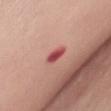biopsy status: catalogued during a skin exam; not biopsied
patient: female, in their mid- to late 60s
image: 15 mm crop, total-body photography
body site: the chest